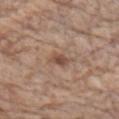| field | value |
|---|---|
| workup | imaged on a skin check; not biopsied |
| diameter | about 3 mm |
| image source | 15 mm crop, total-body photography |
| location | the chest |
| subject | male, in their mid- to late 80s or older |
| lighting | white-light |